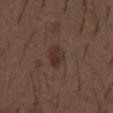Imaged during a routine full-body skin examination; the lesion was not biopsied and no histopathology is available. On the chest. A male subject, aged 48 to 52. An algorithmic analysis of the crop reported an eccentricity of roughly 0.7 and a symmetry-axis asymmetry near 0.3. And it measured border irregularity of about 2.5 on a 0–10 scale. Cropped from a whole-body photographic skin survey; the tile spans about 15 mm. Measured at roughly 3 mm in maximum diameter. Imaged with white-light lighting.